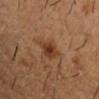Clinical impression: The lesion was tiled from a total-body skin photograph and was not biopsied. Context: A 15 mm close-up extracted from a 3D total-body photography capture. The subject is a male aged 53 to 57. Automated tile analysis of the lesion measured a lesion area of about 6 mm² and two-axis asymmetry of about 0.35. Longest diameter approximately 3.5 mm. On the right upper arm.